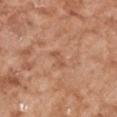Cropped from a total-body skin-imaging series; the visible field is about 15 mm. Imaged with white-light lighting. An algorithmic analysis of the crop reported a footprint of about 2 mm², an eccentricity of roughly 0.9, and a shape-asymmetry score of about 0.5 (0 = symmetric). The analysis additionally found a border-irregularity index near 5.5/10, a within-lesion color-variation index near 0/10, and radial color variation of about 0. Measured at roughly 2.5 mm in maximum diameter. The patient is a female about 75 years old. The lesion is located on the right forearm.